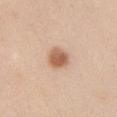Clinical impression:
Captured during whole-body skin photography for melanoma surveillance; the lesion was not biopsied.
Clinical summary:
Measured at roughly 3 mm in maximum diameter. A close-up tile cropped from a whole-body skin photograph, about 15 mm across. Imaged with white-light lighting. A male patient, aged approximately 35. The lesion is located on the left upper arm. An algorithmic analysis of the crop reported a footprint of about 6.5 mm². The analysis additionally found an average lesion color of about L≈61 a*≈21 b*≈33 (CIELAB), a lesion–skin lightness drop of about 13, and a normalized border contrast of about 9.